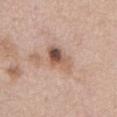Recorded during total-body skin imaging; not selected for excision or biopsy. A female patient, approximately 75 years of age. From the abdomen. A close-up tile cropped from a whole-body skin photograph, about 15 mm across.